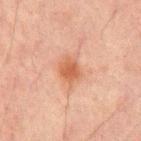No biopsy was performed on this lesion — it was imaged during a full skin examination and was not determined to be concerning. A male subject, aged approximately 65. Cropped from a whole-body photographic skin survey; the tile spans about 15 mm. On the mid back. The lesion-visualizer software estimated an area of roughly 5 mm², an eccentricity of roughly 0.55, and two-axis asymmetry of about 0.15. It also reported a lesion color around L≈47 a*≈22 b*≈31 in CIELAB and roughly 9 lightness units darker than nearby skin.